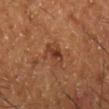<lesion>
<biopsy_status>not biopsied; imaged during a skin examination</biopsy_status>
<patient>
  <sex>male</sex>
  <age_approx>65</age_approx>
</patient>
<lesion_size>
  <long_diameter_mm_approx>3.5</long_diameter_mm_approx>
</lesion_size>
<site>right lower leg</site>
<image>
  <source>total-body photography crop</source>
  <field_of_view_mm>15</field_of_view_mm>
</image>
</lesion>